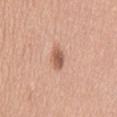A lesion tile, about 15 mm wide, cut from a 3D total-body photograph.
The subject is a female approximately 45 years of age.
From the mid back.
The recorded lesion diameter is about 3 mm.
Automated tile analysis of the lesion measured a footprint of about 4 mm² and a shape-asymmetry score of about 0.3 (0 = symmetric). It also reported a border-irregularity rating of about 3/10 and radial color variation of about 1.
The tile uses white-light illumination.